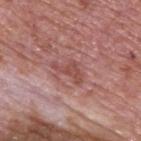The lesion was photographed on a routine skin check and not biopsied; there is no pathology result.